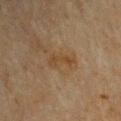Imaged during a routine full-body skin examination; the lesion was not biopsied and no histopathology is available. Imaged with cross-polarized lighting. Located on the right upper arm. Cropped from a whole-body photographic skin survey; the tile spans about 15 mm. A male patient, aged approximately 65.